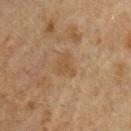Q: Where on the body is the lesion?
A: the arm
Q: What are the patient's age and sex?
A: male, aged around 50
Q: What kind of image is this?
A: ~15 mm tile from a whole-body skin photo
Q: What is the lesion's diameter?
A: ~2.5 mm (longest diameter)